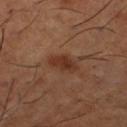Q: Was a biopsy performed?
A: catalogued during a skin exam; not biopsied
Q: What is the lesion's diameter?
A: ~3.5 mm (longest diameter)
Q: What kind of image is this?
A: ~15 mm tile from a whole-body skin photo
Q: Who is the patient?
A: male, approximately 65 years of age
Q: Lesion location?
A: the right thigh
Q: How was the tile lit?
A: cross-polarized illumination